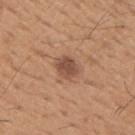<tbp_lesion>
<biopsy_status>not biopsied; imaged during a skin examination</biopsy_status>
<image>
  <source>total-body photography crop</source>
  <field_of_view_mm>15</field_of_view_mm>
</image>
<site>right upper arm</site>
<patient>
  <sex>male</sex>
  <age_approx>65</age_approx>
</patient>
</tbp_lesion>